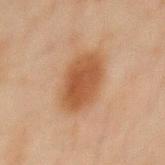{"biopsy_status": "not biopsied; imaged during a skin examination", "site": "mid back", "image": {"source": "total-body photography crop", "field_of_view_mm": 15}, "patient": {"sex": "male", "age_approx": 45}}